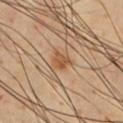| key | value |
|---|---|
| workup | imaged on a skin check; not biopsied |
| subject | male, aged 63–67 |
| location | the chest |
| tile lighting | cross-polarized illumination |
| image | ~15 mm crop, total-body skin-cancer survey |